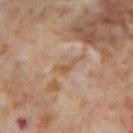No biopsy was performed on this lesion — it was imaged during a full skin examination and was not determined to be concerning.
The lesion is located on the right thigh.
The total-body-photography lesion software estimated a lesion area of about 3 mm², an eccentricity of roughly 0.9, and two-axis asymmetry of about 0.55.
A female subject aged 53 to 57.
Approximately 3 mm at its widest.
A region of skin cropped from a whole-body photographic capture, roughly 15 mm wide.
This is a cross-polarized tile.